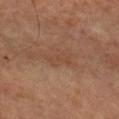Clinical impression:
Recorded during total-body skin imaging; not selected for excision or biopsy.
Background:
The lesion is located on the left forearm. A lesion tile, about 15 mm wide, cut from a 3D total-body photograph. The patient is approximately 65 years of age. Approximately 3 mm at its widest. The lesion-visualizer software estimated a nevus-likeness score of about 0/100 and lesion-presence confidence of about 100/100. This is a cross-polarized tile.